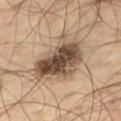Impression: Part of a total-body skin-imaging series; this lesion was reviewed on a skin check and was not flagged for biopsy. Background: Measured at roughly 6.5 mm in maximum diameter. An algorithmic analysis of the crop reported a border-irregularity rating of about 4/10, a within-lesion color-variation index near 7.5/10, and a peripheral color-asymmetry measure near 2.5. It also reported a lesion-detection confidence of about 100/100. A male patient, roughly 55 years of age. Located on the right thigh. A 15 mm close-up extracted from a 3D total-body photography capture.